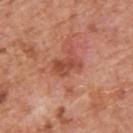Part of a total-body skin-imaging series; this lesion was reviewed on a skin check and was not flagged for biopsy. A male subject, roughly 65 years of age. From the upper back. A 15 mm crop from a total-body photograph taken for skin-cancer surveillance. The recorded lesion diameter is about 3.5 mm.